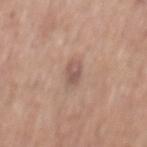<lesion>
  <biopsy_status>not biopsied; imaged during a skin examination</biopsy_status>
  <patient>
    <sex>male</sex>
    <age_approx>60</age_approx>
  </patient>
  <lesion_size>
    <long_diameter_mm_approx>3.0</long_diameter_mm_approx>
  </lesion_size>
  <image>
    <source>total-body photography crop</source>
    <field_of_view_mm>15</field_of_view_mm>
  </image>
  <lighting>white-light</lighting>
  <site>back</site>
  <automated_metrics>
    <shape_asymmetry>0.15</shape_asymmetry>
    <nevus_likeness_0_100>0</nevus_likeness_0_100>
    <lesion_detection_confidence_0_100>100</lesion_detection_confidence_0_100>
  </automated_metrics>
</lesion>